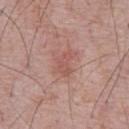Clinical impression: The lesion was photographed on a routine skin check and not biopsied; there is no pathology result. Clinical summary: A region of skin cropped from a whole-body photographic capture, roughly 15 mm wide. A male patient, approximately 60 years of age. Located on the chest.